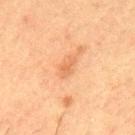| key | value |
|---|---|
| notes | total-body-photography surveillance lesion; no biopsy |
| lighting | cross-polarized illumination |
| imaging modality | ~15 mm crop, total-body skin-cancer survey |
| TBP lesion metrics | an average lesion color of about L≈51 a*≈22 b*≈34 (CIELAB), a lesion–skin lightness drop of about 7, and a normalized border contrast of about 5; a border-irregularity index near 3/10, a within-lesion color-variation index near 0.5/10, and peripheral color asymmetry of about 0 |
| site | the mid back |
| patient | male, aged around 50 |
| size | ~2.5 mm (longest diameter) |